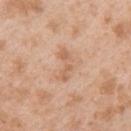{
  "biopsy_status": "not biopsied; imaged during a skin examination",
  "patient": {
    "sex": "male",
    "age_approx": 25
  },
  "image": {
    "source": "total-body photography crop",
    "field_of_view_mm": 15
  },
  "site": "upper back"
}